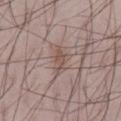Case summary:
• notes · imaged on a skin check; not biopsied
• tile lighting · white-light
• image · 15 mm crop, total-body photography
• site · the left thigh
• subject · male, approximately 40 years of age
• diameter · about 3 mm